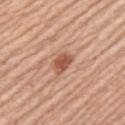follow-up — catalogued during a skin exam; not biopsied
subject — male, in their mid-60s
site — the left upper arm
image source — ~15 mm crop, total-body skin-cancer survey